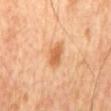Clinical impression:
The lesion was photographed on a routine skin check and not biopsied; there is no pathology result.
Background:
A roughly 15 mm field-of-view crop from a total-body skin photograph. The subject is a male aged 58–62. Imaged with cross-polarized lighting. The lesion is located on the back. The lesion-visualizer software estimated a footprint of about 6.5 mm² and an eccentricity of roughly 0.8. It also reported a border-irregularity index near 2.5/10, internal color variation of about 2.5 on a 0–10 scale, and peripheral color asymmetry of about 0.5. And it measured a lesion-detection confidence of about 100/100.